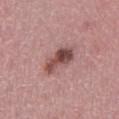Findings:
* follow-up: catalogued during a skin exam; not biopsied
* tile lighting: white-light
* body site: the left lower leg
* image: total-body-photography crop, ~15 mm field of view
* diameter: about 5 mm
* patient: female, about 45 years old